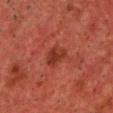notes: catalogued during a skin exam; not biopsied | subject: male, aged 48 to 52 | imaging modality: ~15 mm tile from a whole-body skin photo | site: the chest.